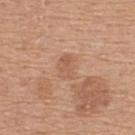workup=imaged on a skin check; not biopsied
image=total-body-photography crop, ~15 mm field of view
site=the upper back
patient=female, roughly 60 years of age